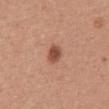Q: Is there a histopathology result?
A: catalogued during a skin exam; not biopsied
Q: What is the lesion's diameter?
A: about 2.5 mm
Q: What is the anatomic site?
A: the mid back
Q: Automated lesion metrics?
A: an average lesion color of about L≈50 a*≈24 b*≈31 (CIELAB), a lesion–skin lightness drop of about 13, and a lesion-to-skin contrast of about 9 (normalized; higher = more distinct)
Q: What lighting was used for the tile?
A: white-light illumination
Q: Who is the patient?
A: male, aged 43 to 47
Q: What kind of image is this?
A: ~15 mm crop, total-body skin-cancer survey